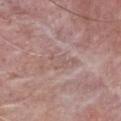The lesion was tiled from a total-body skin photograph and was not biopsied. The subject is a male aged 63–67. Imaged with white-light lighting. The total-body-photography lesion software estimated an area of roughly 2.5 mm², an eccentricity of roughly 0.9, and a symmetry-axis asymmetry near 0.45. And it measured a border-irregularity index near 5/10, internal color variation of about 0 on a 0–10 scale, and a peripheral color-asymmetry measure near 0. And it measured a nevus-likeness score of about 0/100. A 15 mm close-up tile from a total-body photography series done for melanoma screening. Measured at roughly 2.5 mm in maximum diameter. From the chest.